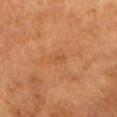Q: Was this lesion biopsied?
A: catalogued during a skin exam; not biopsied
Q: Illumination type?
A: cross-polarized
Q: Patient demographics?
A: female, approximately 65 years of age
Q: How was this image acquired?
A: ~15 mm crop, total-body skin-cancer survey
Q: Lesion location?
A: the head or neck